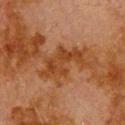| feature | finding |
|---|---|
| follow-up | imaged on a skin check; not biopsied |
| subject | male, in their 80s |
| illumination | cross-polarized |
| anatomic site | the upper back |
| automated lesion analysis | an eccentricity of roughly 0.9 and two-axis asymmetry of about 0.5; a mean CIELAB color near L≈33 a*≈21 b*≈31 and a lesion–skin lightness drop of about 7; border irregularity of about 8 on a 0–10 scale and peripheral color asymmetry of about 1 |
| size | ~6.5 mm (longest diameter) |
| image source | total-body-photography crop, ~15 mm field of view |